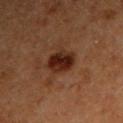Assessment: No biopsy was performed on this lesion — it was imaged during a full skin examination and was not determined to be concerning. Background: The lesion is located on the right upper arm. A close-up tile cropped from a whole-body skin photograph, about 15 mm across. The subject is a male about 60 years old. Longest diameter approximately 4 mm.